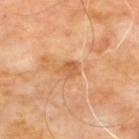Clinical impression: This lesion was catalogued during total-body skin photography and was not selected for biopsy. Image and clinical context: A 15 mm crop from a total-body photograph taken for skin-cancer surveillance. Approximately 2.5 mm at its widest. A male patient aged 63–67. Located on the upper back. Imaged with cross-polarized lighting.